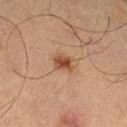No biopsy was performed on this lesion — it was imaged during a full skin examination and was not determined to be concerning. This image is a 15 mm lesion crop taken from a total-body photograph. The lesion-visualizer software estimated a footprint of about 4.5 mm², an eccentricity of roughly 0.65, and a symmetry-axis asymmetry near 0.25. The software also gave an average lesion color of about L≈39 a*≈18 b*≈29 (CIELAB), a lesion–skin lightness drop of about 10, and a normalized lesion–skin contrast near 9. From the left thigh. The patient is a male aged around 70. Imaged with cross-polarized lighting.